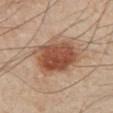patient=male, aged around 50 | lesion diameter=about 7.5 mm | automated lesion analysis=a lesion area of about 24 mm², an eccentricity of roughly 0.8, and a symmetry-axis asymmetry near 0.2; a nevus-likeness score of about 100/100 | site=the left arm | tile lighting=cross-polarized illumination | acquisition=~15 mm crop, total-body skin-cancer survey.